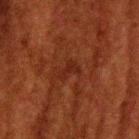{
  "biopsy_status": "not biopsied; imaged during a skin examination",
  "image": {
    "source": "total-body photography crop",
    "field_of_view_mm": 15
  },
  "automated_metrics": {
    "area_mm2_approx": 3.5,
    "eccentricity": 0.8,
    "shape_asymmetry": 0.45,
    "border_irregularity_0_10": 4.5,
    "color_variation_0_10": 0.5,
    "peripheral_color_asymmetry": 0.0,
    "nevus_likeness_0_100": 0,
    "lesion_detection_confidence_0_100": 95
  },
  "site": "head or neck",
  "patient": {
    "sex": "male",
    "age_approx": 60
  },
  "lighting": "cross-polarized"
}